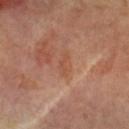Clinical impression:
The lesion was photographed on a routine skin check and not biopsied; there is no pathology result.
Image and clinical context:
Imaged with cross-polarized lighting. The lesion is on the left forearm. Approximately 3 mm at its widest. A male patient approximately 70 years of age. A region of skin cropped from a whole-body photographic capture, roughly 15 mm wide. The lesion-visualizer software estimated a lesion color around L≈49 a*≈23 b*≈30 in CIELAB and a normalized border contrast of about 4.5. And it measured a border-irregularity rating of about 4.5/10 and peripheral color asymmetry of about 0.5.